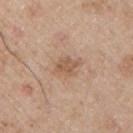  biopsy_status: not biopsied; imaged during a skin examination
  site: left upper arm
  automated_metrics:
    eccentricity: 0.65
    shape_asymmetry: 0.25
    cielab_L: 56
    cielab_a: 18
    cielab_b: 31
    vs_skin_darker_L: 8.0
    vs_skin_contrast_norm: 6.0
    nevus_likeness_0_100: 0
  image:
    source: total-body photography crop
    field_of_view_mm: 15
  patient:
    sex: male
    age_approx: 65
  lesion_size:
    long_diameter_mm_approx: 2.5
  lighting: white-light